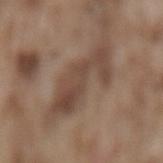This lesion was catalogued during total-body skin photography and was not selected for biopsy. A lesion tile, about 15 mm wide, cut from a 3D total-body photograph. Automated tile analysis of the lesion measured a lesion area of about 19 mm², a shape eccentricity near 0.9, and a shape-asymmetry score of about 0.45 (0 = symmetric). The analysis additionally found a lesion color around L≈45 a*≈16 b*≈25 in CIELAB, a lesion–skin lightness drop of about 9, and a normalized lesion–skin contrast near 7. The subject is a male in their mid-70s. On the lower back. Measured at roughly 7.5 mm in maximum diameter.